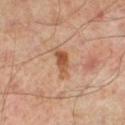workup = no biopsy performed (imaged during a skin exam)
image source = 15 mm crop, total-body photography
illumination = cross-polarized illumination
subject = male, aged around 50
diameter = ~4 mm (longest diameter)
body site = the left lower leg